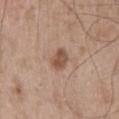* biopsy status · no biopsy performed (imaged during a skin exam)
* site · the back
* patient · male, aged around 50
* imaging modality · ~15 mm tile from a whole-body skin photo
* diameter · about 2.5 mm
* tile lighting · white-light illumination
* automated metrics · an eccentricity of roughly 0.6 and two-axis asymmetry of about 0.25; a mean CIELAB color near L≈51 a*≈19 b*≈29 and a lesion–skin lightness drop of about 11; a border-irregularity index near 2/10, a within-lesion color-variation index near 2/10, and peripheral color asymmetry of about 0.5